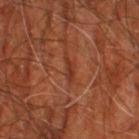Captured during whole-body skin photography for melanoma surveillance; the lesion was not biopsied. A male subject, about 60 years old. A roughly 15 mm field-of-view crop from a total-body skin photograph. The lesion is on the leg.